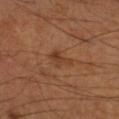{
  "biopsy_status": "not biopsied; imaged during a skin examination",
  "site": "right lower leg",
  "patient": {
    "sex": "male",
    "age_approx": 55
  },
  "lesion_size": {
    "long_diameter_mm_approx": 3.0
  },
  "image": {
    "source": "total-body photography crop",
    "field_of_view_mm": 15
  }
}